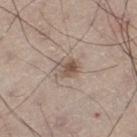{
  "biopsy_status": "not biopsied; imaged during a skin examination",
  "site": "left thigh",
  "lighting": "white-light",
  "patient": {
    "sex": "male",
    "age_approx": 60
  },
  "image": {
    "source": "total-body photography crop",
    "field_of_view_mm": 15
  },
  "automated_metrics": {
    "area_mm2_approx": 4.0,
    "eccentricity": 0.7,
    "shape_asymmetry": 0.3,
    "cielab_L": 53,
    "cielab_a": 14,
    "cielab_b": 24,
    "vs_skin_darker_L": 11.0,
    "vs_skin_contrast_norm": 7.5,
    "lesion_detection_confidence_0_100": 100
  }
}